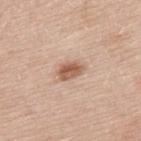workup: no biopsy performed (imaged during a skin exam)
patient: male, roughly 65 years of age
image: ~15 mm tile from a whole-body skin photo
diameter: ≈3.5 mm
tile lighting: white-light
anatomic site: the upper back